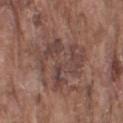Q: Is there a histopathology result?
A: total-body-photography surveillance lesion; no biopsy
Q: What are the patient's age and sex?
A: male, in their mid- to late 70s
Q: What kind of image is this?
A: ~15 mm crop, total-body skin-cancer survey
Q: How large is the lesion?
A: ~6.5 mm (longest diameter)
Q: Lesion location?
A: the right upper arm
Q: Automated lesion metrics?
A: a footprint of about 25 mm², an outline eccentricity of about 0.6 (0 = round, 1 = elongated), and two-axis asymmetry of about 0.45; a mean CIELAB color near L≈43 a*≈18 b*≈21, about 7 CIELAB-L* units darker than the surrounding skin, and a normalized border contrast of about 6.5; border irregularity of about 8 on a 0–10 scale and radial color variation of about 1.5